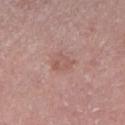workup = imaged on a skin check; not biopsied | location = the leg | diameter = ~2.5 mm (longest diameter) | image source = ~15 mm tile from a whole-body skin photo | automated lesion analysis = an average lesion color of about L≈56 a*≈21 b*≈24 (CIELAB) and about 6 CIELAB-L* units darker than the surrounding skin; border irregularity of about 2.5 on a 0–10 scale and peripheral color asymmetry of about 1; a nevus-likeness score of about 0/100 | subject = male, aged around 75.